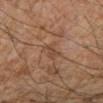| field | value |
|---|---|
| follow-up | catalogued during a skin exam; not biopsied |
| tile lighting | cross-polarized |
| body site | the arm |
| lesion size | ≈3 mm |
| patient | female, approximately 45 years of age |
| image | total-body-photography crop, ~15 mm field of view |